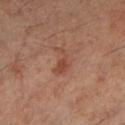notes = imaged on a skin check; not biopsied | site = the left lower leg | lesion size = ≈3.5 mm | tile lighting = cross-polarized illumination | image source = ~15 mm tile from a whole-body skin photo | patient = male, aged around 60.